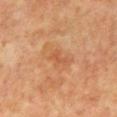Q: Was a biopsy performed?
A: imaged on a skin check; not biopsied
Q: Who is the patient?
A: male, aged 58–62
Q: Illumination type?
A: cross-polarized
Q: What is the lesion's diameter?
A: ~3 mm (longest diameter)
Q: Lesion location?
A: the mid back
Q: How was this image acquired?
A: 15 mm crop, total-body photography
Q: What did automated image analysis measure?
A: a border-irregularity index near 5/10 and radial color variation of about 0; a nevus-likeness score of about 0/100 and a lesion-detection confidence of about 100/100